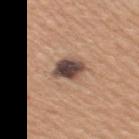biopsy status: catalogued during a skin exam; not biopsied | anatomic site: the right upper arm | size: about 4 mm | patient: female, in their 30s | acquisition: ~15 mm tile from a whole-body skin photo | lighting: white-light | automated lesion analysis: an area of roughly 8.5 mm², an eccentricity of roughly 0.7, and a symmetry-axis asymmetry near 0.25; a detector confidence of about 100 out of 100 that the crop contains a lesion.